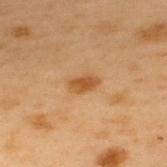Findings:
• workup — no biopsy performed (imaged during a skin exam)
• location — the upper back
• lighting — cross-polarized
• lesion diameter — ~3 mm (longest diameter)
• subject — male, aged around 55
• image source — total-body-photography crop, ~15 mm field of view